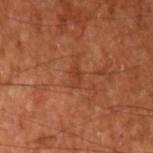| key | value |
|---|---|
| biopsy status | imaged on a skin check; not biopsied |
| body site | the arm |
| image-analysis metrics | a footprint of about 3 mm² and a shape eccentricity near 0.85; a border-irregularity rating of about 4.5/10 and a color-variation rating of about 1/10 |
| imaging modality | ~15 mm crop, total-body skin-cancer survey |
| patient | aged around 65 |
| diameter | ≈2.5 mm |
| lighting | cross-polarized |